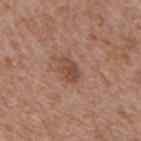Findings:
* notes · total-body-photography surveillance lesion; no biopsy
* size · ~3 mm (longest diameter)
* lighting · white-light
* automated lesion analysis · a lesion color around L≈47 a*≈21 b*≈29 in CIELAB and a lesion-to-skin contrast of about 7 (normalized; higher = more distinct); border irregularity of about 2.5 on a 0–10 scale and peripheral color asymmetry of about 1; an automated nevus-likeness rating near 50 out of 100 and lesion-presence confidence of about 100/100
* image · 15 mm crop, total-body photography
* patient · male, approximately 65 years of age
* anatomic site · the mid back